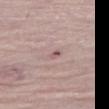A female patient, aged 63 to 67.
Imaged with white-light lighting.
A lesion tile, about 15 mm wide, cut from a 3D total-body photograph.
The total-body-photography lesion software estimated an area of roughly 3 mm², an outline eccentricity of about 0.85 (0 = round, 1 = elongated), and two-axis asymmetry of about 0.3. And it measured a lesion color around L≈59 a*≈17 b*≈19 in CIELAB and roughly 7 lightness units darker than nearby skin. And it measured a peripheral color-asymmetry measure near 1.5. The analysis additionally found an automated nevus-likeness rating near 0 out of 100 and a detector confidence of about 90 out of 100 that the crop contains a lesion.
Located on the left leg.